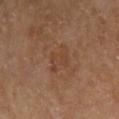biopsy_status: not biopsied; imaged during a skin examination
patient:
  sex: male
  age_approx: 70
site: right upper arm
automated_metrics:
  area_mm2_approx: 5.5
  eccentricity: 0.65
  shape_asymmetry: 0.3
  cielab_L: 41
  cielab_a: 19
  cielab_b: 30
  vs_skin_contrast_norm: 4.5
lesion_size:
  long_diameter_mm_approx: 3.0
image:
  source: total-body photography crop
  field_of_view_mm: 15